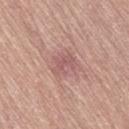Part of a total-body skin-imaging series; this lesion was reviewed on a skin check and was not flagged for biopsy. About 3 mm across. Imaged with white-light lighting. The subject is a male roughly 80 years of age. The lesion is on the right thigh. An algorithmic analysis of the crop reported a within-lesion color-variation index near 1/10 and a peripheral color-asymmetry measure near 0. The analysis additionally found a classifier nevus-likeness of about 0/100 and a lesion-detection confidence of about 100/100. This image is a 15 mm lesion crop taken from a total-body photograph.